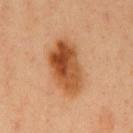{"biopsy_status": "not biopsied; imaged during a skin examination", "image": {"source": "total-body photography crop", "field_of_view_mm": 15}, "patient": {"sex": "male", "age_approx": 50}, "site": "chest"}